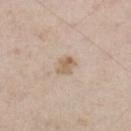Case summary:
* follow-up: no biopsy performed (imaged during a skin exam)